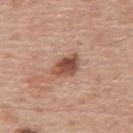<lesion>
<lighting>white-light</lighting>
<site>upper back</site>
<image>
  <source>total-body photography crop</source>
  <field_of_view_mm>15</field_of_view_mm>
</image>
<lesion_size>
  <long_diameter_mm_approx>3.5</long_diameter_mm_approx>
</lesion_size>
<patient>
  <sex>male</sex>
  <age_approx>60</age_approx>
</patient>
</lesion>